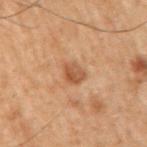Captured during whole-body skin photography for melanoma surveillance; the lesion was not biopsied.
A male subject, aged around 70.
The lesion is on the right upper arm.
Automated tile analysis of the lesion measured lesion-presence confidence of about 100/100.
This image is a 15 mm lesion crop taken from a total-body photograph.
Captured under cross-polarized illumination.
Measured at roughly 3 mm in maximum diameter.